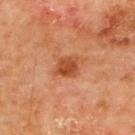The lesion was photographed on a routine skin check and not biopsied; there is no pathology result.
An algorithmic analysis of the crop reported a footprint of about 5.5 mm² and a symmetry-axis asymmetry near 0.25. And it measured border irregularity of about 2 on a 0–10 scale, a color-variation rating of about 3/10, and radial color variation of about 1. And it measured a classifier nevus-likeness of about 95/100 and a lesion-detection confidence of about 100/100.
A male subject, in their mid- to late 60s.
About 3 mm across.
Located on the upper back.
Cropped from a total-body skin-imaging series; the visible field is about 15 mm.
Captured under cross-polarized illumination.